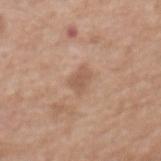{"biopsy_status": "not biopsied; imaged during a skin examination", "image": {"source": "total-body photography crop", "field_of_view_mm": 15}, "site": "abdomen", "automated_metrics": {"area_mm2_approx": 4.0, "eccentricity": 0.8, "cielab_L": 56, "cielab_a": 19, "cielab_b": 29, "border_irregularity_0_10": 3.0, "color_variation_0_10": 1.5, "peripheral_color_asymmetry": 0.5, "nevus_likeness_0_100": 0, "lesion_detection_confidence_0_100": 100}, "lighting": "white-light", "patient": {"sex": "female", "age_approx": 75}, "lesion_size": {"long_diameter_mm_approx": 3.0}}